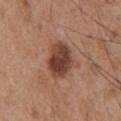Impression:
Captured during whole-body skin photography for melanoma surveillance; the lesion was not biopsied.
Image and clinical context:
A close-up tile cropped from a whole-body skin photograph, about 15 mm across. The subject is a male in their mid-50s. This is a white-light tile. Located on the chest. About 4.5 mm across.